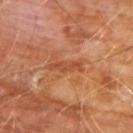follow-up: no biopsy performed (imaged during a skin exam) | automated lesion analysis: a footprint of about 4.5 mm², an outline eccentricity of about 0.95 (0 = round, 1 = elongated), and two-axis asymmetry of about 0.3 | image source: ~15 mm crop, total-body skin-cancer survey | lighting: cross-polarized | lesion size: ≈4 mm | body site: the chest | subject: male, aged 58 to 62.